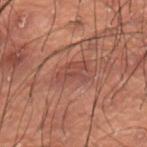| key | value |
|---|---|
| workup | imaged on a skin check; not biopsied |
| subject | male, aged 48–52 |
| anatomic site | the lower back |
| image | 15 mm crop, total-body photography |
| diameter | about 4 mm |